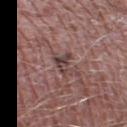Clinical impression:
Captured during whole-body skin photography for melanoma surveillance; the lesion was not biopsied.
Image and clinical context:
An algorithmic analysis of the crop reported a footprint of about 5 mm² and an outline eccentricity of about 0.8 (0 = round, 1 = elongated). It also reported a mean CIELAB color near L≈42 a*≈18 b*≈18, roughly 9 lightness units darker than nearby skin, and a normalized border contrast of about 7.5. It also reported a border-irregularity index near 5.5/10, a color-variation rating of about 4/10, and peripheral color asymmetry of about 1.5. And it measured a nevus-likeness score of about 0/100 and a lesion-detection confidence of about 90/100. The lesion is located on the right thigh. A 15 mm close-up extracted from a 3D total-body photography capture. A male patient aged approximately 60.